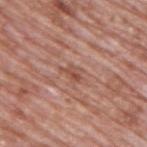The lesion was tiled from a total-body skin photograph and was not biopsied.
A male patient about 70 years old.
The lesion is located on the upper back.
The lesion-visualizer software estimated an area of roughly 3.5 mm². And it measured a mean CIELAB color near L≈51 a*≈24 b*≈28 and a normalized border contrast of about 6. It also reported a border-irregularity rating of about 4/10, internal color variation of about 4 on a 0–10 scale, and peripheral color asymmetry of about 1.5. And it measured a classifier nevus-likeness of about 0/100 and a lesion-detection confidence of about 85/100.
A region of skin cropped from a whole-body photographic capture, roughly 15 mm wide.
Captured under white-light illumination.